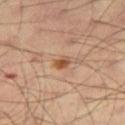This lesion was catalogued during total-body skin photography and was not selected for biopsy. This is a cross-polarized tile. Longest diameter approximately 2 mm. A male subject, roughly 65 years of age. Cropped from a total-body skin-imaging series; the visible field is about 15 mm. The lesion is located on the right thigh.